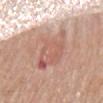Clinical impression: The lesion was tiled from a total-body skin photograph and was not biopsied. Context: The subject is a female aged 68 to 72. Captured under white-light illumination. From the back. About 6 mm across. A region of skin cropped from a whole-body photographic capture, roughly 15 mm wide.